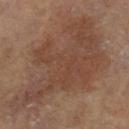workup: total-body-photography surveillance lesion; no biopsy | imaging modality: ~15 mm tile from a whole-body skin photo | site: the right lower leg | patient: female, roughly 75 years of age | diameter: about 14 mm | tile lighting: cross-polarized illumination.